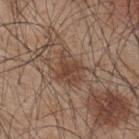notes: catalogued during a skin exam; not biopsied
size: about 3.5 mm
imaging modality: ~15 mm tile from a whole-body skin photo
tile lighting: white-light
subject: male, aged around 45
body site: the upper back